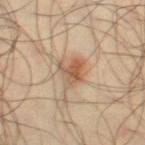The lesion was tiled from a total-body skin photograph and was not biopsied. From the right thigh. A male patient in their mid-40s. The tile uses cross-polarized illumination. A 15 mm close-up tile from a total-body photography series done for melanoma screening.